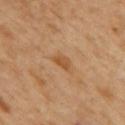biopsy_status: not biopsied; imaged during a skin examination
lesion_size:
  long_diameter_mm_approx: 2.5
image:
  source: total-body photography crop
  field_of_view_mm: 15
patient:
  sex: male
  age_approx: 50
site: mid back